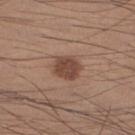Clinical summary: Captured under white-light illumination. About 3 mm across. A male patient, in their mid-30s. A 15 mm crop from a total-body photograph taken for skin-cancer surveillance. The lesion is located on the right lower leg.